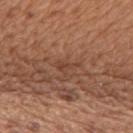Q: Was this lesion biopsied?
A: catalogued during a skin exam; not biopsied
Q: What kind of image is this?
A: ~15 mm crop, total-body skin-cancer survey
Q: Who is the patient?
A: male, aged 63–67
Q: What did automated image analysis measure?
A: an automated nevus-likeness rating near 0 out of 100 and lesion-presence confidence of about 55/100
Q: Where on the body is the lesion?
A: the mid back
Q: Illumination type?
A: white-light illumination